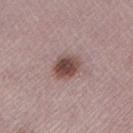patient — female, aged 48 to 52 | anatomic site — the left lower leg | image — 15 mm crop, total-body photography.